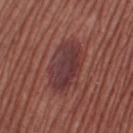Q: Was a biopsy performed?
A: no biopsy performed (imaged during a skin exam)